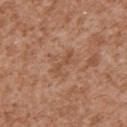Longest diameter approximately 3.5 mm. A 15 mm crop from a total-body photograph taken for skin-cancer surveillance. The tile uses white-light illumination. The subject is a male about 45 years old. The lesion is located on the left upper arm. The total-body-photography lesion software estimated a lesion area of about 3 mm², an eccentricity of roughly 0.95, and two-axis asymmetry of about 0.5. And it measured about 7 CIELAB-L* units darker than the surrounding skin and a normalized lesion–skin contrast near 5.5. And it measured border irregularity of about 6 on a 0–10 scale and a peripheral color-asymmetry measure near 0. It also reported an automated nevus-likeness rating near 0 out of 100.